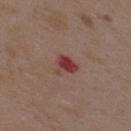| feature | finding |
|---|---|
| biopsy status | catalogued during a skin exam; not biopsied |
| tile lighting | white-light |
| image-analysis metrics | an area of roughly 5 mm², an outline eccentricity of about 0.4 (0 = round, 1 = elongated), and a shape-asymmetry score of about 0.45 (0 = symmetric); a lesion-detection confidence of about 100/100 |
| site | the upper back |
| imaging modality | 15 mm crop, total-body photography |
| subject | female, roughly 35 years of age |